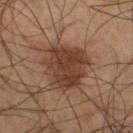Q: Is there a histopathology result?
A: imaged on a skin check; not biopsied
Q: Who is the patient?
A: male, aged 58 to 62
Q: How was the tile lit?
A: cross-polarized illumination
Q: How large is the lesion?
A: ≈6 mm
Q: What did automated image analysis measure?
A: a mean CIELAB color near L≈37 a*≈19 b*≈28 and a normalized lesion–skin contrast near 9; border irregularity of about 3.5 on a 0–10 scale, a color-variation rating of about 3.5/10, and a peripheral color-asymmetry measure near 1; a nevus-likeness score of about 50/100
Q: Where on the body is the lesion?
A: the right thigh
Q: How was this image acquired?
A: 15 mm crop, total-body photography